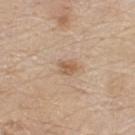biopsy_status: not biopsied; imaged during a skin examination
site: back
lighting: white-light
automated_metrics:
  vs_skin_contrast_norm: 7.0
  border_irregularity_0_10: 3.0
  color_variation_0_10: 2.5
  peripheral_color_asymmetry: 1.0
  nevus_likeness_0_100: 45
  lesion_detection_confidence_0_100: 100
patient:
  sex: male
  age_approx: 80
image:
  source: total-body photography crop
  field_of_view_mm: 15